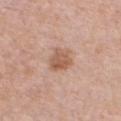patient — female, aged approximately 40; body site — the chest; acquisition — ~15 mm tile from a whole-body skin photo; lesion size — ≈3.5 mm; lighting — white-light.